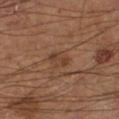- biopsy status · no biopsy performed (imaged during a skin exam)
- location · the right leg
- illumination · cross-polarized illumination
- image · total-body-photography crop, ~15 mm field of view
- patient · male, aged 58–62